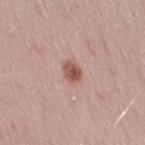biopsy_status: not biopsied; imaged during a skin examination
automated_metrics:
  area_mm2_approx: 4.0
  nevus_likeness_0_100: 95
  lesion_detection_confidence_0_100: 100
lighting: white-light
lesion_size:
  long_diameter_mm_approx: 2.5
site: right thigh
patient:
  sex: female
  age_approx: 30
image:
  source: total-body photography crop
  field_of_view_mm: 15